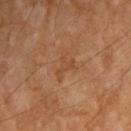Part of a total-body skin-imaging series; this lesion was reviewed on a skin check and was not flagged for biopsy.
An algorithmic analysis of the crop reported an eccentricity of roughly 0.8 and a shape-asymmetry score of about 0.65 (0 = symmetric). It also reported roughly 5 lightness units darker than nearby skin and a lesion-to-skin contrast of about 5 (normalized; higher = more distinct).
About 2.5 mm across.
On the right upper arm.
A male subject, approximately 55 years of age.
A 15 mm close-up tile from a total-body photography series done for melanoma screening.